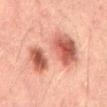Context:
A male subject, roughly 50 years of age. The recorded lesion diameter is about 9 mm. Captured under cross-polarized illumination. Cropped from a whole-body photographic skin survey; the tile spans about 15 mm. On the mid back. The total-body-photography lesion software estimated a lesion area of about 32 mm², an outline eccentricity of about 0.85 (0 = round, 1 = elongated), and two-axis asymmetry of about 0.5. It also reported a lesion-to-skin contrast of about 8 (normalized; higher = more distinct). The software also gave lesion-presence confidence of about 100/100.
Diagnosis:
Histopathology of the biopsied lesion showed a dysplastic (Clark) nevus.